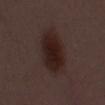Q: Was a biopsy performed?
A: no biopsy performed (imaged during a skin exam)
Q: What lighting was used for the tile?
A: white-light illumination
Q: Who is the patient?
A: male, in their 50s
Q: What did automated image analysis measure?
A: a footprint of about 18 mm², an eccentricity of roughly 0.85, and two-axis asymmetry of about 0.15; a lesion–skin lightness drop of about 9 and a normalized border contrast of about 11; an automated nevus-likeness rating near 100 out of 100 and lesion-presence confidence of about 100/100
Q: How large is the lesion?
A: ≈6.5 mm
Q: What is the imaging modality?
A: ~15 mm crop, total-body skin-cancer survey